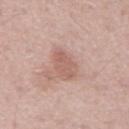The lesion was tiled from a total-body skin photograph and was not biopsied.
Approximately 3.5 mm at its widest.
A male patient, approximately 55 years of age.
The lesion-visualizer software estimated a shape eccentricity near 0.8 and two-axis asymmetry of about 0.25. It also reported a lesion–skin lightness drop of about 8 and a normalized lesion–skin contrast near 5.5. It also reported a border-irregularity index near 2.5/10 and a peripheral color-asymmetry measure near 0.5.
This image is a 15 mm lesion crop taken from a total-body photograph.
Located on the mid back.